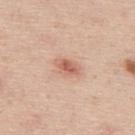Image and clinical context:
A 15 mm crop from a total-body photograph taken for skin-cancer surveillance. A male patient, aged 43–47. The lesion is located on the back.